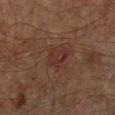<lesion>
<biopsy_status>not biopsied; imaged during a skin examination</biopsy_status>
<image>
  <source>total-body photography crop</source>
  <field_of_view_mm>15</field_of_view_mm>
</image>
<site>left lower leg</site>
<lesion_size>
  <long_diameter_mm_approx>3.5</long_diameter_mm_approx>
</lesion_size>
<patient>
  <sex>male</sex>
  <age_approx>60</age_approx>
</patient>
</lesion>